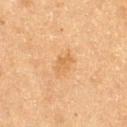Q: Was this lesion biopsied?
A: catalogued during a skin exam; not biopsied
Q: Lesion location?
A: the leg
Q: What is the imaging modality?
A: ~15 mm crop, total-body skin-cancer survey
Q: Who is the patient?
A: female, aged around 55